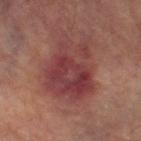Clinical impression:
Imaged during a routine full-body skin examination; the lesion was not biopsied and no histopathology is available.
Acquisition and patient details:
From the right lower leg. The recorded lesion diameter is about 7.5 mm. Automated tile analysis of the lesion measured a detector confidence of about 100 out of 100 that the crop contains a lesion. This is a cross-polarized tile. A male patient, about 70 years old. A roughly 15 mm field-of-view crop from a total-body skin photograph.